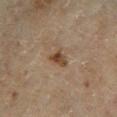Findings:
• follow-up — total-body-photography surveillance lesion; no biopsy
• patient — male, in their mid-60s
• diameter — about 2.5 mm
• image — ~15 mm tile from a whole-body skin photo
• site — the right lower leg
• lighting — cross-polarized illumination